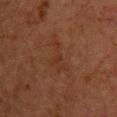Q: What is the imaging modality?
A: 15 mm crop, total-body photography
Q: Lesion location?
A: the chest
Q: What are the patient's age and sex?
A: female, aged approximately 50